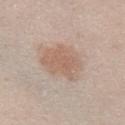Q: Was this lesion biopsied?
A: imaged on a skin check; not biopsied
Q: What is the anatomic site?
A: the chest
Q: What are the patient's age and sex?
A: female, aged 38–42
Q: Automated lesion metrics?
A: an average lesion color of about L≈61 a*≈17 b*≈27 (CIELAB) and a normalized lesion–skin contrast near 6; an automated nevus-likeness rating near 50 out of 100 and a detector confidence of about 100 out of 100 that the crop contains a lesion
Q: What is the imaging modality?
A: ~15 mm crop, total-body skin-cancer survey
Q: Lesion size?
A: about 5 mm
Q: What lighting was used for the tile?
A: white-light illumination